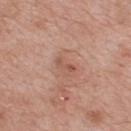Acquisition and patient details:
A male patient about 65 years old. The lesion-visualizer software estimated a footprint of about 2 mm², an outline eccentricity of about 0.95 (0 = round, 1 = elongated), and two-axis asymmetry of about 0.5. The software also gave a mean CIELAB color near L≈53 a*≈23 b*≈28, roughly 8 lightness units darker than nearby skin, and a lesion-to-skin contrast of about 6 (normalized; higher = more distinct). It also reported a classifier nevus-likeness of about 0/100 and a lesion-detection confidence of about 100/100. Located on the upper back. A 15 mm close-up extracted from a 3D total-body photography capture. The lesion's longest dimension is about 2.5 mm. This is a white-light tile.